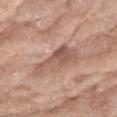From the right forearm. Captured under white-light illumination. A female subject, in their mid-70s. The total-body-photography lesion software estimated a shape-asymmetry score of about 0.5 (0 = symmetric). The analysis additionally found an average lesion color of about L≈55 a*≈20 b*≈26 (CIELAB), a lesion–skin lightness drop of about 11, and a normalized lesion–skin contrast near 7. The software also gave a border-irregularity index near 5.5/10. The software also gave an automated nevus-likeness rating near 0 out of 100. A lesion tile, about 15 mm wide, cut from a 3D total-body photograph. Longest diameter approximately 5.5 mm.